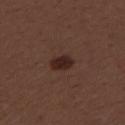Background: From the upper back. This is a white-light tile. The recorded lesion diameter is about 3 mm. An algorithmic analysis of the crop reported an average lesion color of about L≈25 a*≈18 b*≈20 (CIELAB), a lesion–skin lightness drop of about 9, and a lesion-to-skin contrast of about 10 (normalized; higher = more distinct). And it measured a classifier nevus-likeness of about 90/100 and a lesion-detection confidence of about 100/100. The patient is a female about 50 years old. A 15 mm close-up tile from a total-body photography series done for melanoma screening.